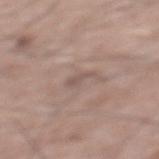{"biopsy_status": "not biopsied; imaged during a skin examination", "image": {"source": "total-body photography crop", "field_of_view_mm": 15}, "site": "mid back", "patient": {"sex": "male", "age_approx": 60}}